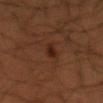Q: Was a biopsy performed?
A: catalogued during a skin exam; not biopsied
Q: What did automated image analysis measure?
A: a lesion area of about 3.5 mm², a shape eccentricity near 0.8, and two-axis asymmetry of about 0.35; a nevus-likeness score of about 90/100
Q: What are the patient's age and sex?
A: male, roughly 50 years of age
Q: What is the imaging modality?
A: ~15 mm tile from a whole-body skin photo
Q: How large is the lesion?
A: ≈2.5 mm
Q: Lesion location?
A: the arm
Q: How was the tile lit?
A: cross-polarized illumination